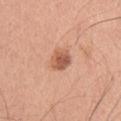Clinical impression:
The lesion was tiled from a total-body skin photograph and was not biopsied.
Image and clinical context:
Automated image analysis of the tile measured a lesion-to-skin contrast of about 8 (normalized; higher = more distinct). The software also gave border irregularity of about 2 on a 0–10 scale and a within-lesion color-variation index near 3/10. Captured under white-light illumination. A male subject aged 58–62. A 15 mm crop from a total-body photograph taken for skin-cancer surveillance. Located on the chest. Approximately 3 mm at its widest.